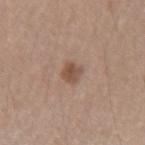  biopsy_status: not biopsied; imaged during a skin examination
  lighting: white-light
  site: right forearm
  image:
    source: total-body photography crop
    field_of_view_mm: 15
  patient:
    sex: female
    age_approx: 45
  lesion_size:
    long_diameter_mm_approx: 2.5